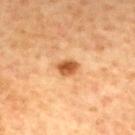Imaged during a routine full-body skin examination; the lesion was not biopsied and no histopathology is available. A close-up tile cropped from a whole-body skin photograph, about 15 mm across. The lesion is on the upper back. The subject is a male aged approximately 60.